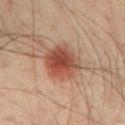Captured during whole-body skin photography for melanoma surveillance; the lesion was not biopsied. Captured under cross-polarized illumination. From the front of the torso. A 15 mm close-up tile from a total-body photography series done for melanoma screening. An algorithmic analysis of the crop reported a footprint of about 14 mm², a shape eccentricity near 0.5, and a shape-asymmetry score of about 0.15 (0 = symmetric). It also reported a mean CIELAB color near L≈50 a*≈25 b*≈31 and a lesion-to-skin contrast of about 9 (normalized; higher = more distinct). And it measured a border-irregularity index near 2/10, a color-variation rating of about 7.5/10, and a peripheral color-asymmetry measure near 2.5. A male subject about 40 years old.